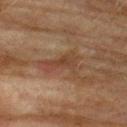Recorded during total-body skin imaging; not selected for excision or biopsy.
A 15 mm close-up extracted from a 3D total-body photography capture.
Imaged with cross-polarized lighting.
The subject is a male aged approximately 75.
The lesion is located on the upper back.